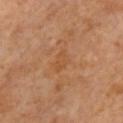Q: Is there a histopathology result?
A: no biopsy performed (imaged during a skin exam)
Q: What kind of image is this?
A: 15 mm crop, total-body photography
Q: How was the tile lit?
A: cross-polarized
Q: What is the lesion's diameter?
A: ~3 mm (longest diameter)
Q: Lesion location?
A: the chest
Q: Who is the patient?
A: male, about 60 years old
Q: What did automated image analysis measure?
A: an outline eccentricity of about 0.8 (0 = round, 1 = elongated) and a symmetry-axis asymmetry near 0.35; an average lesion color of about L≈49 a*≈22 b*≈37 (CIELAB) and a normalized lesion–skin contrast near 4.5